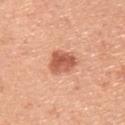Imaged during a routine full-body skin examination; the lesion was not biopsied and no histopathology is available.
This is a white-light tile.
The recorded lesion diameter is about 4 mm.
On the back.
A male subject, aged 43 to 47.
A roughly 15 mm field-of-view crop from a total-body skin photograph.